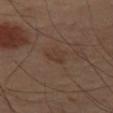From the left thigh. The lesion's longest dimension is about 2.5 mm. A lesion tile, about 15 mm wide, cut from a 3D total-body photograph. Captured under cross-polarized illumination.